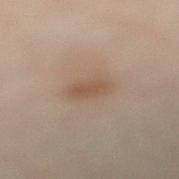{"biopsy_status": "not biopsied; imaged during a skin examination", "image": {"source": "total-body photography crop", "field_of_view_mm": 15}, "lesion_size": {"long_diameter_mm_approx": 3.0}, "lighting": "cross-polarized", "site": "left leg", "patient": {"sex": "male", "age_approx": 50}}